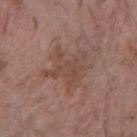The lesion was tiled from a total-body skin photograph and was not biopsied.
A region of skin cropped from a whole-body photographic capture, roughly 15 mm wide.
Approximately 5 mm at its widest.
A male subject, aged approximately 60.
The total-body-photography lesion software estimated a lesion color around L≈46 a*≈18 b*≈24 in CIELAB, roughly 6 lightness units darker than nearby skin, and a normalized border contrast of about 5. It also reported a border-irregularity index near 8/10, a color-variation rating of about 2.5/10, and radial color variation of about 1.
Located on the right forearm.
This is a white-light tile.